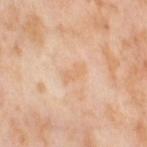<lesion>
  <biopsy_status>not biopsied; imaged during a skin examination</biopsy_status>
  <site>right thigh</site>
  <automated_metrics>
    <eccentricity>0.85</eccentricity>
    <shape_asymmetry>0.3</shape_asymmetry>
    <border_irregularity_0_10>3.5</border_irregularity_0_10>
    <peripheral_color_asymmetry>0.5</peripheral_color_asymmetry>
    <nevus_likeness_0_100>0</nevus_likeness_0_100>
    <lesion_detection_confidence_0_100>100</lesion_detection_confidence_0_100>
  </automated_metrics>
  <lighting>cross-polarized</lighting>
  <patient>
    <sex>female</sex>
    <age_approx>55</age_approx>
  </patient>
  <image>
    <source>total-body photography crop</source>
    <field_of_view_mm>15</field_of_view_mm>
  </image>
</lesion>